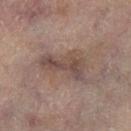Assessment:
Captured during whole-body skin photography for melanoma surveillance; the lesion was not biopsied.
Clinical summary:
A female patient aged approximately 80. Captured under cross-polarized illumination. A region of skin cropped from a whole-body photographic capture, roughly 15 mm wide. The lesion is on the left leg. The lesion's longest dimension is about 6 mm. Automated image analysis of the tile measured an area of roughly 13 mm² and a shape-asymmetry score of about 0.45 (0 = symmetric). The software also gave a lesion-detection confidence of about 90/100.